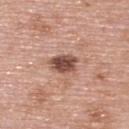Clinical impression:
This lesion was catalogued during total-body skin photography and was not selected for biopsy.
Context:
This is a white-light tile. From the upper back. The total-body-photography lesion software estimated a footprint of about 7 mm², an outline eccentricity of about 0.7 (0 = round, 1 = elongated), and a shape-asymmetry score of about 0.15 (0 = symmetric). It also reported a mean CIELAB color near L≈49 a*≈22 b*≈26 and a normalized border contrast of about 11. The software also gave border irregularity of about 2 on a 0–10 scale, a color-variation rating of about 3.5/10, and a peripheral color-asymmetry measure near 1. About 3.5 mm across. A lesion tile, about 15 mm wide, cut from a 3D total-body photograph. The subject is a female aged 58 to 62.